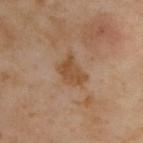• follow-up · total-body-photography surveillance lesion; no biopsy
• subject · male, roughly 55 years of age
• size · ≈3.5 mm
• lighting · cross-polarized illumination
• site · the back
• automated metrics · an area of roughly 6.5 mm² and two-axis asymmetry of about 0.35; an average lesion color of about L≈49 a*≈19 b*≈34 (CIELAB), a lesion–skin lightness drop of about 9, and a normalized border contrast of about 8; a nevus-likeness score of about 0/100 and lesion-presence confidence of about 100/100
• image source · 15 mm crop, total-body photography